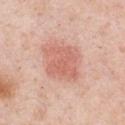follow-up = catalogued during a skin exam; not biopsied
diameter = ≈4.5 mm
TBP lesion metrics = a lesion-detection confidence of about 100/100
location = the chest
image source = ~15 mm crop, total-body skin-cancer survey
illumination = white-light illumination
patient = male, in their 50s